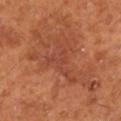Clinical impression:
The lesion was tiled from a total-body skin photograph and was not biopsied.
Acquisition and patient details:
Located on the leg. This image is a 15 mm lesion crop taken from a total-body photograph. A male patient, approximately 65 years of age. Automated image analysis of the tile measured an area of roughly 8.5 mm² and a shape-asymmetry score of about 0.7 (0 = symmetric). The analysis additionally found a mean CIELAB color near L≈45 a*≈29 b*≈32 and about 6 CIELAB-L* units darker than the surrounding skin. It also reported a border-irregularity rating of about 10/10 and internal color variation of about 2.5 on a 0–10 scale. This is a cross-polarized tile. Measured at roughly 5.5 mm in maximum diameter.